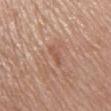Assessment: No biopsy was performed on this lesion — it was imaged during a full skin examination and was not determined to be concerning. Clinical summary: A female subject in their mid-60s. The lesion is located on the back. The lesion's longest dimension is about 3 mm. The lesion-visualizer software estimated a mean CIELAB color near L≈54 a*≈23 b*≈29 and a lesion-to-skin contrast of about 5.5 (normalized; higher = more distinct). The software also gave a classifier nevus-likeness of about 0/100 and lesion-presence confidence of about 95/100. A 15 mm crop from a total-body photograph taken for skin-cancer surveillance. This is a white-light tile.